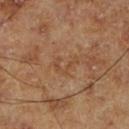  biopsy_status: not biopsied; imaged during a skin examination
  site: right lower leg
  image:
    source: total-body photography crop
    field_of_view_mm: 15
  automated_metrics:
    eccentricity: 0.8
    shape_asymmetry: 0.7
    cielab_L: 45
    cielab_a: 20
    cielab_b: 33
    vs_skin_darker_L: 5.0
    border_irregularity_0_10: 10.0
    color_variation_0_10: 0.0
    peripheral_color_asymmetry: 0.0
    nevus_likeness_0_100: 0
  lighting: cross-polarized
  lesion_size:
    long_diameter_mm_approx: 3.0
  patient:
    sex: male
    age_approx: 70